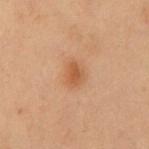Impression: Part of a total-body skin-imaging series; this lesion was reviewed on a skin check and was not flagged for biopsy. Clinical summary: Located on the chest. The patient is a male in their mid- to late 50s. A roughly 15 mm field-of-view crop from a total-body skin photograph.